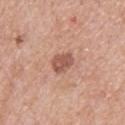image:
  source: total-body photography crop
  field_of_view_mm: 15
site: upper back
automated_metrics:
  area_mm2_approx: 5.0
  eccentricity: 0.55
  shape_asymmetry: 0.25
  cielab_L: 54
  cielab_a: 23
  cielab_b: 29
  vs_skin_darker_L: 11.0
  border_irregularity_0_10: 2.5
  color_variation_0_10: 2.5
  peripheral_color_asymmetry: 1.0
lighting: white-light
lesion_size:
  long_diameter_mm_approx: 3.0
patient:
  sex: male
  age_approx: 60